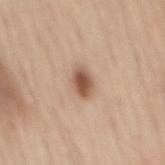Context: This image is a 15 mm lesion crop taken from a total-body photograph. The subject is a male approximately 65 years of age. The total-body-photography lesion software estimated a footprint of about 5.5 mm² and a shape eccentricity near 0.7. And it measured a mean CIELAB color near L≈55 a*≈19 b*≈29, a lesion–skin lightness drop of about 14, and a lesion-to-skin contrast of about 9 (normalized; higher = more distinct). And it measured a classifier nevus-likeness of about 95/100 and lesion-presence confidence of about 100/100. From the mid back. The lesion's longest dimension is about 3 mm.